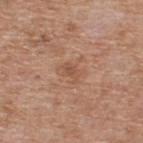Recorded during total-body skin imaging; not selected for excision or biopsy.
A male patient roughly 60 years of age.
The lesion is located on the upper back.
A 15 mm close-up extracted from a 3D total-body photography capture.